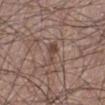Findings:
• workup: no biopsy performed (imaged during a skin exam)